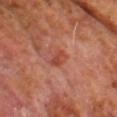follow-up: imaged on a skin check; not biopsied
patient: male, roughly 60 years of age
diameter: about 2.5 mm
lighting: cross-polarized
image source: ~15 mm tile from a whole-body skin photo
anatomic site: the back